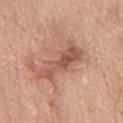Context:
Cropped from a total-body skin-imaging series; the visible field is about 15 mm. An algorithmic analysis of the crop reported a mean CIELAB color near L≈56 a*≈23 b*≈28, a lesion–skin lightness drop of about 10, and a normalized lesion–skin contrast near 7. It also reported a border-irregularity rating of about 5.5/10, a within-lesion color-variation index near 5/10, and a peripheral color-asymmetry measure near 1.5. And it measured lesion-presence confidence of about 100/100. The tile uses white-light illumination. The patient is a male aged around 75. Longest diameter approximately 7 mm. From the chest.